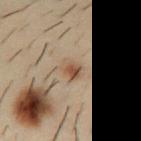Findings:
– notes · catalogued during a skin exam; not biopsied
– tile lighting · cross-polarized
– lesion size · ≈2.5 mm
– subject · male, aged around 30
– anatomic site · the front of the torso
– image · 15 mm crop, total-body photography